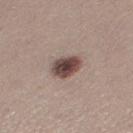Captured during whole-body skin photography for melanoma surveillance; the lesion was not biopsied.
A region of skin cropped from a whole-body photographic capture, roughly 15 mm wide.
The subject is a female roughly 25 years of age.
The recorded lesion diameter is about 3.5 mm.
Located on the left thigh.